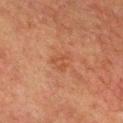Imaged during a routine full-body skin examination; the lesion was not biopsied and no histopathology is available. A 15 mm close-up tile from a total-body photography series done for melanoma screening. The recorded lesion diameter is about 3 mm. A male patient, in their 60s. From the head or neck. This is a cross-polarized tile.